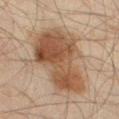Q: Was this lesion biopsied?
A: no biopsy performed (imaged during a skin exam)
Q: Lesion location?
A: the left thigh
Q: Automated lesion metrics?
A: an area of roughly 37 mm², an eccentricity of roughly 0.8, and two-axis asymmetry of about 0.3; an average lesion color of about L≈41 a*≈15 b*≈27 (CIELAB), about 11 CIELAB-L* units darker than the surrounding skin, and a lesion-to-skin contrast of about 9.5 (normalized; higher = more distinct)
Q: How was this image acquired?
A: ~15 mm tile from a whole-body skin photo
Q: Illumination type?
A: cross-polarized
Q: Who is the patient?
A: male, roughly 45 years of age
Q: What is the lesion's diameter?
A: ~9 mm (longest diameter)